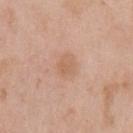Impression:
The lesion was photographed on a routine skin check and not biopsied; there is no pathology result.
Acquisition and patient details:
Automated image analysis of the tile measured a lesion area of about 5.5 mm², a shape eccentricity near 0.6, and a symmetry-axis asymmetry near 0.25. It also reported a classifier nevus-likeness of about 5/100 and a lesion-detection confidence of about 100/100. The lesion's longest dimension is about 3 mm. A close-up tile cropped from a whole-body skin photograph, about 15 mm across. On the arm. A female patient, aged 38 to 42.